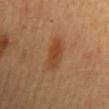Captured during whole-body skin photography for melanoma surveillance; the lesion was not biopsied. The tile uses cross-polarized illumination. On the mid back. The subject is a female aged 58 to 62. Longest diameter approximately 4.5 mm. A 15 mm close-up extracted from a 3D total-body photography capture. The total-body-photography lesion software estimated a lesion area of about 8 mm², a shape eccentricity near 0.9, and a symmetry-axis asymmetry near 0.2. And it measured a border-irregularity index near 3/10, internal color variation of about 3.5 on a 0–10 scale, and a peripheral color-asymmetry measure near 1. The analysis additionally found a classifier nevus-likeness of about 95/100 and a lesion-detection confidence of about 100/100.